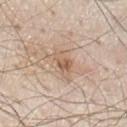Case summary:
– follow-up · total-body-photography surveillance lesion; no biopsy
– imaging modality · total-body-photography crop, ~15 mm field of view
– size · ~4 mm (longest diameter)
– automated metrics · a footprint of about 5.5 mm² and an outline eccentricity of about 0.85 (0 = round, 1 = elongated); a color-variation rating of about 5.5/10 and a peripheral color-asymmetry measure near 2
– subject · male, aged 78 to 82
– location · the chest